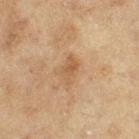Q: What are the patient's age and sex?
A: female, aged 53–57
Q: Automated lesion metrics?
A: a lesion area of about 5 mm², an outline eccentricity of about 0.8 (0 = round, 1 = elongated), and a shape-asymmetry score of about 0.3 (0 = symmetric); border irregularity of about 3.5 on a 0–10 scale, internal color variation of about 2.5 on a 0–10 scale, and peripheral color asymmetry of about 1
Q: What lighting was used for the tile?
A: cross-polarized illumination
Q: What is the lesion's diameter?
A: about 3.5 mm
Q: Lesion location?
A: the left thigh
Q: What is the imaging modality?
A: ~15 mm crop, total-body skin-cancer survey